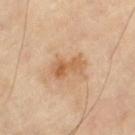<lesion>
  <biopsy_status>not biopsied; imaged during a skin examination</biopsy_status>
  <patient>
    <sex>male</sex>
    <age_approx>65</age_approx>
  </patient>
  <image>
    <source>total-body photography crop</source>
    <field_of_view_mm>15</field_of_view_mm>
  </image>
  <site>left thigh</site>
</lesion>